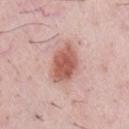Context: Automated image analysis of the tile measured a lesion color around L≈58 a*≈25 b*≈27 in CIELAB and a lesion–skin lightness drop of about 14. The analysis additionally found border irregularity of about 2 on a 0–10 scale and internal color variation of about 4 on a 0–10 scale. A lesion tile, about 15 mm wide, cut from a 3D total-body photograph. Imaged with white-light lighting. Located on the chest. The lesion's longest dimension is about 5 mm. A male subject roughly 40 years of age.